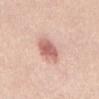Notes:
* biopsy status — no biopsy performed (imaged during a skin exam)
* subject — male, roughly 40 years of age
* body site — the abdomen
* size — ~5.5 mm (longest diameter)
* acquisition — total-body-photography crop, ~15 mm field of view
* tile lighting — white-light